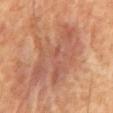Impression:
No biopsy was performed on this lesion — it was imaged during a full skin examination and was not determined to be concerning.
Image and clinical context:
Approximately 10 mm at its widest. This is a cross-polarized tile. An algorithmic analysis of the crop reported a footprint of about 31 mm², an eccentricity of roughly 0.85, and a shape-asymmetry score of about 0.4 (0 = symmetric). Located on the chest. A 15 mm close-up extracted from a 3D total-body photography capture. The patient is a male approximately 60 years of age.